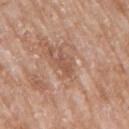Impression: This lesion was catalogued during total-body skin photography and was not selected for biopsy. Clinical summary: A female patient about 75 years old. Located on the right upper arm. Cropped from a total-body skin-imaging series; the visible field is about 15 mm.